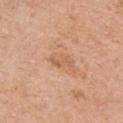Impression:
Captured during whole-body skin photography for melanoma surveillance; the lesion was not biopsied.
Clinical summary:
A male patient, in their 70s. The lesion's longest dimension is about 3 mm. The lesion is on the front of the torso. A 15 mm crop from a total-body photograph taken for skin-cancer surveillance.